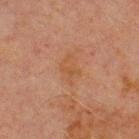Q: Is there a histopathology result?
A: total-body-photography surveillance lesion; no biopsy
Q: Lesion size?
A: ≈3.5 mm
Q: What lighting was used for the tile?
A: cross-polarized
Q: What kind of image is this?
A: total-body-photography crop, ~15 mm field of view
Q: Patient demographics?
A: male, approximately 70 years of age
Q: What is the anatomic site?
A: the chest